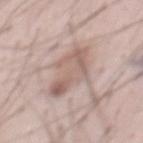Clinical impression: The lesion was tiled from a total-body skin photograph and was not biopsied. Acquisition and patient details: This image is a 15 mm lesion crop taken from a total-body photograph. The lesion is located on the front of the torso. The patient is a male approximately 55 years of age. This is a white-light tile. The lesion-visualizer software estimated a lesion color around L≈61 a*≈16 b*≈23 in CIELAB, about 10 CIELAB-L* units darker than the surrounding skin, and a normalized lesion–skin contrast near 6.5. It also reported a nevus-likeness score of about 0/100 and a detector confidence of about 45 out of 100 that the crop contains a lesion. Measured at roughly 6.5 mm in maximum diameter.